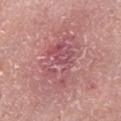- workup — no biopsy performed (imaged during a skin exam)